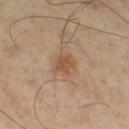Part of a total-body skin-imaging series; this lesion was reviewed on a skin check and was not flagged for biopsy.
A male patient in their mid-50s.
Cropped from a whole-body photographic skin survey; the tile spans about 15 mm.
The lesion is on the leg.
Measured at roughly 3 mm in maximum diameter.
Imaged with cross-polarized lighting.